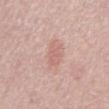| key | value |
|---|---|
| follow-up | catalogued during a skin exam; not biopsied |
| acquisition | ~15 mm crop, total-body skin-cancer survey |
| location | the abdomen |
| patient | male, aged around 70 |